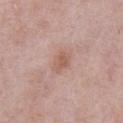follow-up = imaged on a skin check; not biopsied | subject = male, roughly 55 years of age | image source = ~15 mm tile from a whole-body skin photo | anatomic site = the abdomen.